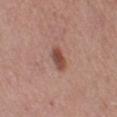biopsy status — no biopsy performed (imaged during a skin exam); tile lighting — white-light; patient — female, approximately 30 years of age; imaging modality — total-body-photography crop, ~15 mm field of view; size — about 3 mm; site — the right thigh.